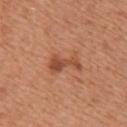The lesion was photographed on a routine skin check and not biopsied; there is no pathology result. Measured at roughly 4 mm in maximum diameter. The total-body-photography lesion software estimated a lesion–skin lightness drop of about 10 and a lesion-to-skin contrast of about 7 (normalized; higher = more distinct). The software also gave a nevus-likeness score of about 40/100 and a detector confidence of about 100 out of 100 that the crop contains a lesion. A female patient, approximately 50 years of age. A 15 mm close-up tile from a total-body photography series done for melanoma screening. Captured under white-light illumination. From the right upper arm.